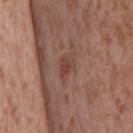Q: Is there a histopathology result?
A: no biopsy performed (imaged during a skin exam)
Q: Lesion size?
A: about 3 mm
Q: Patient demographics?
A: male, aged around 45
Q: Where on the body is the lesion?
A: the front of the torso
Q: How was this image acquired?
A: ~15 mm crop, total-body skin-cancer survey
Q: What lighting was used for the tile?
A: white-light illumination